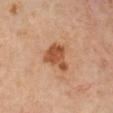Clinical impression:
The lesion was tiled from a total-body skin photograph and was not biopsied.
Context:
A female subject aged approximately 50. On the left lower leg. Captured under cross-polarized illumination. A roughly 15 mm field-of-view crop from a total-body skin photograph. Longest diameter approximately 3.5 mm.